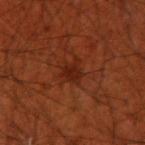Assessment: Captured during whole-body skin photography for melanoma surveillance; the lesion was not biopsied. Acquisition and patient details: Cropped from a whole-body photographic skin survey; the tile spans about 15 mm. The subject is a male in their 70s. On the right upper arm.